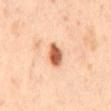Part of a total-body skin-imaging series; this lesion was reviewed on a skin check and was not flagged for biopsy.
A male patient, aged 63–67.
A roughly 15 mm field-of-view crop from a total-body skin photograph.
The lesion's longest dimension is about 3.5 mm.
Automated tile analysis of the lesion measured an outline eccentricity of about 0.8 (0 = round, 1 = elongated). It also reported roughly 19 lightness units darker than nearby skin and a lesion-to-skin contrast of about 11 (normalized; higher = more distinct). It also reported a nevus-likeness score of about 100/100.
From the mid back.